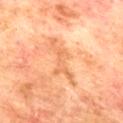<record>
  <biopsy_status>not biopsied; imaged during a skin examination</biopsy_status>
  <lesion_size>
    <long_diameter_mm_approx>6.0</long_diameter_mm_approx>
  </lesion_size>
  <image>
    <source>total-body photography crop</source>
    <field_of_view_mm>15</field_of_view_mm>
  </image>
  <patient>
    <sex>male</sex>
    <age_approx>75</age_approx>
  </patient>
  <lighting>cross-polarized</lighting>
  <site>mid back</site>
  <automated_metrics>
    <lesion_detection_confidence_0_100>95</lesion_detection_confidence_0_100>
  </automated_metrics>
</record>